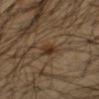notes — imaged on a skin check; not biopsied
image-analysis metrics — a within-lesion color-variation index near 3.5/10 and a peripheral color-asymmetry measure near 1; a classifier nevus-likeness of about 80/100 and a detector confidence of about 100 out of 100 that the crop contains a lesion
illumination — cross-polarized
acquisition — total-body-photography crop, ~15 mm field of view
subject — male, aged approximately 45
location — the arm